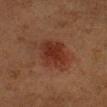Recorded during total-body skin imaging; not selected for excision or biopsy.
About 4 mm across.
Captured under cross-polarized illumination.
A female subject, aged around 40.
A roughly 15 mm field-of-view crop from a total-body skin photograph.
From the head or neck.